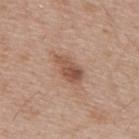The lesion was photographed on a routine skin check and not biopsied; there is no pathology result.
A lesion tile, about 15 mm wide, cut from a 3D total-body photograph.
The patient is a male in their mid- to late 50s.